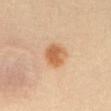Assessment:
Recorded during total-body skin imaging; not selected for excision or biopsy.
Background:
A female subject, aged around 25. The lesion is located on the abdomen. This image is a 15 mm lesion crop taken from a total-body photograph. This is a cross-polarized tile. Automated tile analysis of the lesion measured a lesion color around L≈60 a*≈22 b*≈38 in CIELAB and about 11 CIELAB-L* units darker than the surrounding skin. And it measured border irregularity of about 1 on a 0–10 scale, a within-lesion color-variation index near 4/10, and peripheral color asymmetry of about 1. The analysis additionally found a detector confidence of about 100 out of 100 that the crop contains a lesion.